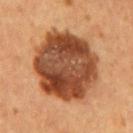Recorded during total-body skin imaging; not selected for excision or biopsy. Automated image analysis of the tile measured a footprint of about 50 mm² and an eccentricity of roughly 0.65. It also reported a border-irregularity index near 1.5/10 and internal color variation of about 8 on a 0–10 scale. And it measured an automated nevus-likeness rating near 35 out of 100. A 15 mm close-up tile from a total-body photography series done for melanoma screening. Located on the mid back. Measured at roughly 10 mm in maximum diameter. A male subject, approximately 55 years of age. The tile uses cross-polarized illumination.